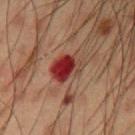Assessment:
Recorded during total-body skin imaging; not selected for excision or biopsy.
Context:
A close-up tile cropped from a whole-body skin photograph, about 15 mm across. From the right upper arm. A male patient, aged 53–57.